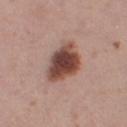{
  "biopsy_status": "not biopsied; imaged during a skin examination",
  "patient": {
    "sex": "female",
    "age_approx": 40
  },
  "lighting": "white-light",
  "lesion_size": {
    "long_diameter_mm_approx": 5.5
  },
  "image": {
    "source": "total-body photography crop",
    "field_of_view_mm": 15
  },
  "site": "right thigh"
}